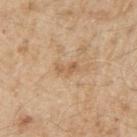Image and clinical context: This is a white-light tile. Approximately 2.5 mm at its widest. This image is a 15 mm lesion crop taken from a total-body photograph. The total-body-photography lesion software estimated a border-irregularity rating of about 4.5/10, internal color variation of about 0 on a 0–10 scale, and a peripheral color-asymmetry measure near 0. And it measured an automated nevus-likeness rating near 0 out of 100. Located on the right upper arm. The subject is a male aged approximately 70.